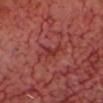Acquisition and patient details:
Measured at roughly 2.5 mm in maximum diameter. From the head or neck. The total-body-photography lesion software estimated a lesion color around L≈35 a*≈32 b*≈27 in CIELAB, a lesion–skin lightness drop of about 6, and a normalized border contrast of about 6. The analysis additionally found a border-irregularity index near 4.5/10, internal color variation of about 3 on a 0–10 scale, and peripheral color asymmetry of about 0.5. A patient roughly 65 years of age. A 15 mm crop from a total-body photograph taken for skin-cancer surveillance. The tile uses cross-polarized illumination.